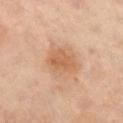Captured during whole-body skin photography for melanoma surveillance; the lesion was not biopsied. The subject is a male roughly 65 years of age. Imaged with cross-polarized lighting. Longest diameter approximately 3.5 mm. A lesion tile, about 15 mm wide, cut from a 3D total-body photograph. The lesion is on the right upper arm.